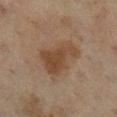workup: imaged on a skin check; not biopsied
patient: female, in their mid- to late 50s
image: total-body-photography crop, ~15 mm field of view
diameter: ~5.5 mm (longest diameter)
location: the right lower leg
illumination: cross-polarized illumination